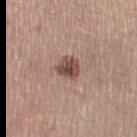- workup · no biopsy performed (imaged during a skin exam)
- acquisition · ~15 mm crop, total-body skin-cancer survey
- tile lighting · white-light
- patient · female, approximately 45 years of age
- body site · the left thigh
- lesion size · ~3 mm (longest diameter)